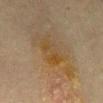notes = total-body-photography surveillance lesion; no biopsy
size = ~4 mm (longest diameter)
automated metrics = a lesion color around L≈39 a*≈13 b*≈33 in CIELAB, a lesion–skin lightness drop of about 4, and a normalized border contrast of about 6.5; a nevus-likeness score of about 0/100 and a lesion-detection confidence of about 100/100
image = ~15 mm crop, total-body skin-cancer survey
patient = male, approximately 75 years of age
illumination = cross-polarized illumination
body site = the mid back